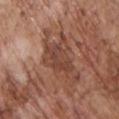<lesion>
<biopsy_status>not biopsied; imaged during a skin examination</biopsy_status>
<image>
  <source>total-body photography crop</source>
  <field_of_view_mm>15</field_of_view_mm>
</image>
<patient>
  <sex>male</sex>
  <age_approx>70</age_approx>
</patient>
<site>chest</site>
</lesion>